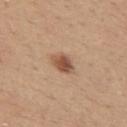Part of a total-body skin-imaging series; this lesion was reviewed on a skin check and was not flagged for biopsy.
From the upper back.
The patient is a female in their mid-40s.
This is a white-light tile.
The lesion-visualizer software estimated a shape eccentricity near 0.7 and a shape-asymmetry score of about 0.2 (0 = symmetric). And it measured a lesion color around L≈52 a*≈20 b*≈31 in CIELAB and a normalized lesion–skin contrast near 9. The software also gave a border-irregularity index near 2/10 and a within-lesion color-variation index near 4.5/10.
A close-up tile cropped from a whole-body skin photograph, about 15 mm across.